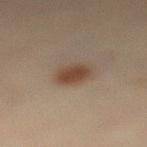biopsy status=imaged on a skin check; not biopsied
anatomic site=the left lower leg
patient=female, in their mid-40s
image source=15 mm crop, total-body photography